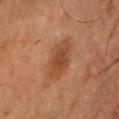Q: What is the anatomic site?
A: the chest
Q: Patient demographics?
A: male, in their mid-50s
Q: What did automated image analysis measure?
A: an area of roughly 12 mm², a shape eccentricity near 0.9, and a symmetry-axis asymmetry near 0.2; roughly 9 lightness units darker than nearby skin; an automated nevus-likeness rating near 70 out of 100 and lesion-presence confidence of about 100/100
Q: How was this image acquired?
A: total-body-photography crop, ~15 mm field of view
Q: How large is the lesion?
A: ≈6 mm